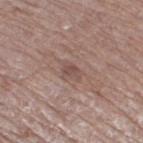No biopsy was performed on this lesion — it was imaged during a full skin examination and was not determined to be concerning. An algorithmic analysis of the crop reported a footprint of about 3 mm². The analysis additionally found a border-irregularity rating of about 3.5/10 and peripheral color asymmetry of about 0.5. Located on the right thigh. A male subject aged 78–82. A close-up tile cropped from a whole-body skin photograph, about 15 mm across. Captured under white-light illumination. Measured at roughly 2.5 mm in maximum diameter.